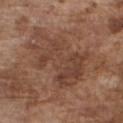notes=imaged on a skin check; not biopsied | tile lighting=white-light illumination | automated lesion analysis=an area of roughly 26 mm² and a symmetry-axis asymmetry near 0.3; a mean CIELAB color near L≈42 a*≈19 b*≈27 and about 7 CIELAB-L* units darker than the surrounding skin; a nevus-likeness score of about 0/100 | location=the front of the torso | subject=male, aged 73–77 | image=15 mm crop, total-body photography | diameter=≈8 mm.